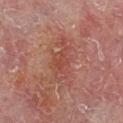notes: catalogued during a skin exam; not biopsied | patient: male, roughly 60 years of age | imaging modality: 15 mm crop, total-body photography | image-analysis metrics: a within-lesion color-variation index near 2.5/10 and peripheral color asymmetry of about 0.5 | site: the right lower leg | lighting: cross-polarized illumination.